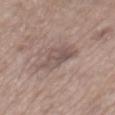This lesion was catalogued during total-body skin photography and was not selected for biopsy.